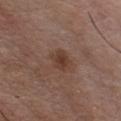Clinical impression:
Captured during whole-body skin photography for melanoma surveillance; the lesion was not biopsied.
Image and clinical context:
Measured at roughly 3 mm in maximum diameter. On the chest. The subject is a male approximately 55 years of age. Cropped from a whole-body photographic skin survey; the tile spans about 15 mm. Imaged with white-light lighting.